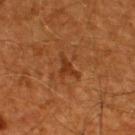Assessment:
Captured during whole-body skin photography for melanoma surveillance; the lesion was not biopsied.
Context:
A male patient aged 58–62. Automated image analysis of the tile measured an automated nevus-likeness rating near 0 out of 100 and a lesion-detection confidence of about 100/100. A roughly 15 mm field-of-view crop from a total-body skin photograph. On the upper back. Captured under cross-polarized illumination. Approximately 3.5 mm at its widest.